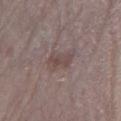Notes:
* notes: catalogued during a skin exam; not biopsied
* TBP lesion metrics: an area of roughly 8 mm² and an outline eccentricity of about 0.6 (0 = round, 1 = elongated); border irregularity of about 2.5 on a 0–10 scale and radial color variation of about 1
* tile lighting: white-light illumination
* subject: male, about 75 years old
* imaging modality: ~15 mm crop, total-body skin-cancer survey
* lesion size: about 3.5 mm
* body site: the right lower leg